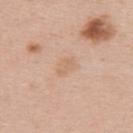Impression:
The lesion was tiled from a total-body skin photograph and was not biopsied.
Image and clinical context:
A female patient, about 35 years old. Imaged with white-light lighting. A roughly 15 mm field-of-view crop from a total-body skin photograph. The lesion is on the upper back.